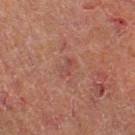<case>
  <biopsy_status>not biopsied; imaged during a skin examination</biopsy_status>
  <lesion_size>
    <long_diameter_mm_approx>2.5</long_diameter_mm_approx>
  </lesion_size>
  <site>left thigh</site>
  <image>
    <source>total-body photography crop</source>
    <field_of_view_mm>15</field_of_view_mm>
  </image>
  <patient>
    <sex>male</sex>
    <age_approx>65</age_approx>
  </patient>
  <lighting>cross-polarized</lighting>
</case>